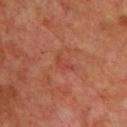<tbp_lesion>
  <biopsy_status>not biopsied; imaged during a skin examination</biopsy_status>
  <site>front of the torso</site>
  <image>
    <source>total-body photography crop</source>
    <field_of_view_mm>15</field_of_view_mm>
  </image>
  <patient>
    <sex>male</sex>
    <age_approx>65</age_approx>
  </patient>
</tbp_lesion>